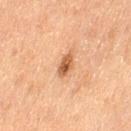The lesion was photographed on a routine skin check and not biopsied; there is no pathology result. Cropped from a whole-body photographic skin survey; the tile spans about 15 mm. A female subject aged 53 to 57. On the left thigh. The total-body-photography lesion software estimated a footprint of about 4 mm², an outline eccentricity of about 0.8 (0 = round, 1 = elongated), and a symmetry-axis asymmetry near 0.3.